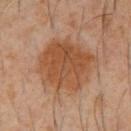The lesion was photographed on a routine skin check and not biopsied; there is no pathology result.
A male subject roughly 60 years of age.
Cropped from a total-body skin-imaging series; the visible field is about 15 mm.
From the chest.
The tile uses cross-polarized illumination.